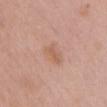Captured during whole-body skin photography for melanoma surveillance; the lesion was not biopsied. Approximately 3.5 mm at its widest. A lesion tile, about 15 mm wide, cut from a 3D total-body photograph. Imaged with white-light lighting. A female patient aged 63 to 67. From the chest. The lesion-visualizer software estimated an area of roughly 6.5 mm², an outline eccentricity of about 0.75 (0 = round, 1 = elongated), and a shape-asymmetry score of about 0.25 (0 = symmetric). And it measured a mean CIELAB color near L≈60 a*≈20 b*≈30, roughly 6 lightness units darker than nearby skin, and a normalized lesion–skin contrast near 5. And it measured a border-irregularity index near 2/10, a within-lesion color-variation index near 3/10, and peripheral color asymmetry of about 1. And it measured a nevus-likeness score of about 0/100 and a detector confidence of about 100 out of 100 that the crop contains a lesion.